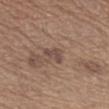No biopsy was performed on this lesion — it was imaged during a full skin examination and was not determined to be concerning. From the arm. The lesion's longest dimension is about 3 mm. A lesion tile, about 15 mm wide, cut from a 3D total-body photograph. A male patient roughly 65 years of age. Captured under white-light illumination.